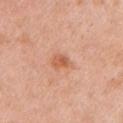Q: Is there a histopathology result?
A: total-body-photography surveillance lesion; no biopsy
Q: What did automated image analysis measure?
A: a border-irregularity rating of about 2/10, a color-variation rating of about 3.5/10, and a peripheral color-asymmetry measure near 1.5; an automated nevus-likeness rating near 55 out of 100 and lesion-presence confidence of about 100/100
Q: What kind of image is this?
A: total-body-photography crop, ~15 mm field of view
Q: How large is the lesion?
A: about 2.5 mm
Q: Patient demographics?
A: female, aged 38 to 42
Q: Lesion location?
A: the left upper arm